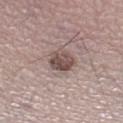biopsy_status: not biopsied; imaged during a skin examination
site: right lower leg
lighting: white-light
image:
  source: total-body photography crop
  field_of_view_mm: 15
patient:
  sex: female
  age_approx: 50
automated_metrics:
  area_mm2_approx: 7.5
  eccentricity: 0.35
  shape_asymmetry: 0.2
  vs_skin_darker_L: 12.0
  vs_skin_contrast_norm: 9.0